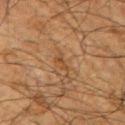Clinical impression: Part of a total-body skin-imaging series; this lesion was reviewed on a skin check and was not flagged for biopsy. Image and clinical context: About 2.5 mm across. The tile uses cross-polarized illumination. An algorithmic analysis of the crop reported an area of roughly 2 mm², an outline eccentricity of about 0.9 (0 = round, 1 = elongated), and a shape-asymmetry score of about 0.45 (0 = symmetric). It also reported an average lesion color of about L≈38 a*≈17 b*≈31 (CIELAB), about 6 CIELAB-L* units darker than the surrounding skin, and a normalized lesion–skin contrast near 6. And it measured a border-irregularity index near 5.5/10, internal color variation of about 0 on a 0–10 scale, and a peripheral color-asymmetry measure near 0. A close-up tile cropped from a whole-body skin photograph, about 15 mm across. On the right upper arm. A male patient, aged 63–67.